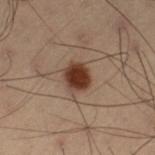No biopsy was performed on this lesion — it was imaged during a full skin examination and was not determined to be concerning. Automated image analysis of the tile measured a mean CIELAB color near L≈29 a*≈16 b*≈23 and a normalized border contrast of about 12.5. The software also gave a nevus-likeness score of about 100/100. Imaged with cross-polarized lighting. A male patient aged around 55. A region of skin cropped from a whole-body photographic capture, roughly 15 mm wide. From the left thigh. Approximately 3.5 mm at its widest.